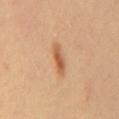biopsy status: catalogued during a skin exam; not biopsied
tile lighting: cross-polarized
patient: female, roughly 50 years of age
image source: 15 mm crop, total-body photography
TBP lesion metrics: a lesion color around L≈58 a*≈23 b*≈37 in CIELAB, roughly 11 lightness units darker than nearby skin, and a lesion-to-skin contrast of about 7.5 (normalized; higher = more distinct); a border-irregularity rating of about 3/10, internal color variation of about 3.5 on a 0–10 scale, and a peripheral color-asymmetry measure near 1; an automated nevus-likeness rating near 95 out of 100 and a detector confidence of about 100 out of 100 that the crop contains a lesion
lesion size: about 4 mm